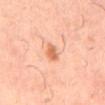The lesion was tiled from a total-body skin photograph and was not biopsied. From the mid back. A region of skin cropped from a whole-body photographic capture, roughly 15 mm wide. Measured at roughly 3 mm in maximum diameter. The patient is a male aged 38–42.